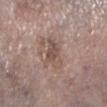Q: Was this lesion biopsied?
A: catalogued during a skin exam; not biopsied
Q: How was this image acquired?
A: ~15 mm crop, total-body skin-cancer survey
Q: What did automated image analysis measure?
A: two-axis asymmetry of about 0.35; a lesion color around L≈51 a*≈16 b*≈24 in CIELAB, a lesion–skin lightness drop of about 9, and a lesion-to-skin contrast of about 6.5 (normalized; higher = more distinct); a border-irregularity index near 4/10, a color-variation rating of about 4.5/10, and a peripheral color-asymmetry measure near 1.5
Q: Patient demographics?
A: female, aged 63 to 67
Q: Where on the body is the lesion?
A: the left lower leg
Q: Lesion size?
A: ~4 mm (longest diameter)
Q: How was the tile lit?
A: white-light illumination